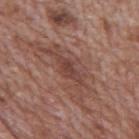workup: total-body-photography surveillance lesion; no biopsy | image: total-body-photography crop, ~15 mm field of view | patient: male, in their 70s | anatomic site: the mid back | TBP lesion metrics: a footprint of about 5 mm² and an outline eccentricity of about 0.95 (0 = round, 1 = elongated) | lesion size: ≈4.5 mm.